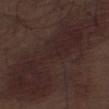Impression: Captured during whole-body skin photography for melanoma surveillance; the lesion was not biopsied. Acquisition and patient details: On the leg. Cropped from a total-body skin-imaging series; the visible field is about 15 mm. The subject is a male in their 70s. About 17 mm across. The tile uses white-light illumination.